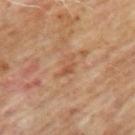{
  "biopsy_status": "not biopsied; imaged during a skin examination",
  "image": {
    "source": "total-body photography crop",
    "field_of_view_mm": 15
  },
  "site": "arm",
  "lighting": "cross-polarized",
  "patient": {
    "sex": "male",
    "age_approx": 65
  }
}